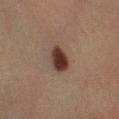Notes:
• subject: female, about 55 years old
• location: the leg
• image source: 15 mm crop, total-body photography